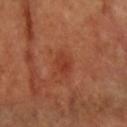Q: Was a biopsy performed?
A: no biopsy performed (imaged during a skin exam)
Q: What lighting was used for the tile?
A: cross-polarized illumination
Q: What is the imaging modality?
A: ~15 mm tile from a whole-body skin photo
Q: What are the patient's age and sex?
A: female, about 60 years old
Q: What is the anatomic site?
A: the left arm
Q: What is the lesion's diameter?
A: about 2.5 mm
Q: What did automated image analysis measure?
A: a nevus-likeness score of about 20/100 and lesion-presence confidence of about 100/100